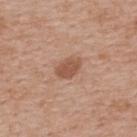<tbp_lesion>
  <lighting>white-light</lighting>
  <lesion_size>
    <long_diameter_mm_approx>3.0</long_diameter_mm_approx>
  </lesion_size>
  <site>upper back</site>
  <image>
    <source>total-body photography crop</source>
    <field_of_view_mm>15</field_of_view_mm>
  </image>
  <patient>
    <sex>male</sex>
    <age_approx>60</age_approx>
  </patient>
</tbp_lesion>